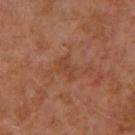Imaged during a routine full-body skin examination; the lesion was not biopsied and no histopathology is available.
Imaged with cross-polarized lighting.
Longest diameter approximately 3 mm.
The lesion is on the left arm.
A lesion tile, about 15 mm wide, cut from a 3D total-body photograph.
A male patient aged 28 to 32.